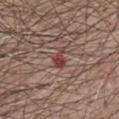The lesion was tiled from a total-body skin photograph and was not biopsied. Located on the chest. A lesion tile, about 15 mm wide, cut from a 3D total-body photograph. This is a white-light tile. The patient is a male approximately 70 years of age.